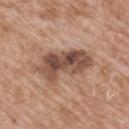No biopsy was performed on this lesion — it was imaged during a full skin examination and was not determined to be concerning.
The lesion is located on the back.
This image is a 15 mm lesion crop taken from a total-body photograph.
The subject is a male roughly 60 years of age.